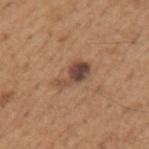Clinical impression: Imaged during a routine full-body skin examination; the lesion was not biopsied and no histopathology is available. Image and clinical context: A male patient aged 63 to 67. Cropped from a whole-body photographic skin survey; the tile spans about 15 mm. Imaged with white-light lighting. From the left upper arm. Automated image analysis of the tile measured an average lesion color of about L≈46 a*≈19 b*≈28 (CIELAB) and a lesion–skin lightness drop of about 12.